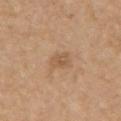Impression:
The lesion was tiled from a total-body skin photograph and was not biopsied.
Context:
An algorithmic analysis of the crop reported a classifier nevus-likeness of about 0/100 and lesion-presence confidence of about 100/100. From the chest. A lesion tile, about 15 mm wide, cut from a 3D total-body photograph. About 2.5 mm across. A male patient, roughly 70 years of age. This is a white-light tile.